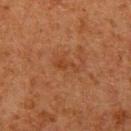• follow-up — no biopsy performed (imaged during a skin exam)
• diameter — ~3 mm (longest diameter)
• acquisition — ~15 mm tile from a whole-body skin photo
• subject — male, aged 78 to 82
• anatomic site — the mid back
• lighting — cross-polarized